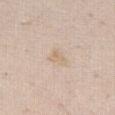biopsy status: catalogued during a skin exam; not biopsied
anatomic site: the right thigh
tile lighting: white-light
subject: female, about 60 years old
image-analysis metrics: a footprint of about 3.5 mm², an eccentricity of roughly 0.75, and a shape-asymmetry score of about 0.4 (0 = symmetric); a lesion color around L≈69 a*≈13 b*≈30 in CIELAB, a lesion–skin lightness drop of about 6, and a normalized lesion–skin contrast near 5; a border-irregularity index near 3.5/10, a within-lesion color-variation index near 2/10, and radial color variation of about 0.5; a classifier nevus-likeness of about 0/100 and lesion-presence confidence of about 100/100
acquisition: ~15 mm crop, total-body skin-cancer survey
diameter: about 2.5 mm